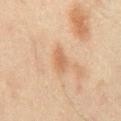<record>
<biopsy_status>not biopsied; imaged during a skin examination</biopsy_status>
<site>abdomen</site>
<image>
  <source>total-body photography crop</source>
  <field_of_view_mm>15</field_of_view_mm>
</image>
<lesion_size>
  <long_diameter_mm_approx>2.5</long_diameter_mm_approx>
</lesion_size>
<lighting>cross-polarized</lighting>
<automated_metrics>
  <area_mm2_approx>3.5</area_mm2_approx>
  <eccentricity>0.85</eccentricity>
  <shape_asymmetry>0.15</shape_asymmetry>
  <cielab_L>50</cielab_L>
  <cielab_a>18</cielab_a>
  <cielab_b>30</cielab_b>
  <vs_skin_darker_L>7.0</vs_skin_darker_L>
  <vs_skin_contrast_norm>6.0</vs_skin_contrast_norm>
  <nevus_likeness_0_100>25</nevus_likeness_0_100>
</automated_metrics>
<patient>
  <sex>male</sex>
  <age_approx>45</age_approx>
</patient>
</record>